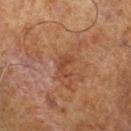follow-up = catalogued during a skin exam; not biopsied | patient = male, roughly 70 years of age | diameter = ~2.5 mm (longest diameter) | location = the right lower leg | acquisition = 15 mm crop, total-body photography | image-analysis metrics = an average lesion color of about L≈36 a*≈20 b*≈28 (CIELAB), about 6 CIELAB-L* units darker than the surrounding skin, and a normalized border contrast of about 6; a border-irregularity rating of about 6/10, internal color variation of about 0 on a 0–10 scale, and radial color variation of about 0; a classifier nevus-likeness of about 0/100 and a lesion-detection confidence of about 100/100.